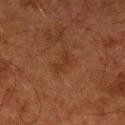follow-up = no biopsy performed (imaged during a skin exam)
automated metrics = a lesion area of about 3 mm², a shape eccentricity near 0.9, and two-axis asymmetry of about 0.45; a mean CIELAB color near L≈26 a*≈19 b*≈26, a lesion–skin lightness drop of about 4, and a normalized lesion–skin contrast near 5; border irregularity of about 6 on a 0–10 scale, internal color variation of about 0 on a 0–10 scale, and peripheral color asymmetry of about 0
lesion diameter = ≈3 mm
anatomic site = the left lower leg
lighting = cross-polarized
patient = male, aged around 80
image = ~15 mm crop, total-body skin-cancer survey